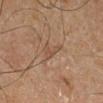This is a cross-polarized tile. Automated image analysis of the tile measured a footprint of about 3.5 mm² and a symmetry-axis asymmetry near 0.55. The software also gave an average lesion color of about L≈39 a*≈14 b*≈24 (CIELAB), about 5 CIELAB-L* units darker than the surrounding skin, and a lesion-to-skin contrast of about 5 (normalized; higher = more distinct). The analysis additionally found a nevus-likeness score of about 0/100 and lesion-presence confidence of about 70/100. A 15 mm crop from a total-body photograph taken for skin-cancer surveillance. A male subject approximately 65 years of age. From the right lower leg.